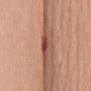biopsy status: total-body-photography surveillance lesion; no biopsy
image: ~15 mm tile from a whole-body skin photo
image-analysis metrics: a lesion area of about 4.5 mm², an eccentricity of roughly 0.85, and a shape-asymmetry score of about 0.25 (0 = symmetric); a mean CIELAB color near L≈48 a*≈27 b*≈25, a lesion–skin lightness drop of about 12, and a lesion-to-skin contrast of about 9 (normalized; higher = more distinct)
lighting: white-light illumination
size: ≈3 mm
subject: female, in their 60s
location: the mid back